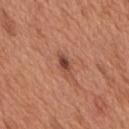Imaged during a routine full-body skin examination; the lesion was not biopsied and no histopathology is available.
The lesion is located on the mid back.
A male subject in their mid-60s.
An algorithmic analysis of the crop reported a lesion area of about 3 mm², an outline eccentricity of about 0.8 (0 = round, 1 = elongated), and two-axis asymmetry of about 0.2. The software also gave roughly 12 lightness units darker than nearby skin.
Imaged with white-light lighting.
Cropped from a total-body skin-imaging series; the visible field is about 15 mm.